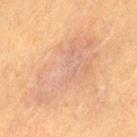Impression:
Recorded during total-body skin imaging; not selected for excision or biopsy.
Context:
A close-up tile cropped from a whole-body skin photograph, about 15 mm across. The lesion is located on the left thigh. A female patient, in their mid-50s.